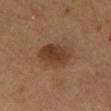follow-up: catalogued during a skin exam; not biopsied | image source: ~15 mm tile from a whole-body skin photo | body site: the mid back | TBP lesion metrics: an automated nevus-likeness rating near 90 out of 100 and a detector confidence of about 100 out of 100 that the crop contains a lesion | subject: male, aged 53 to 57 | lighting: cross-polarized.